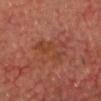follow-up: total-body-photography surveillance lesion; no biopsy | tile lighting: cross-polarized illumination | anatomic site: the head or neck | subject: male, aged 58 to 62 | diameter: ~4 mm (longest diameter) | image source: ~15 mm tile from a whole-body skin photo.